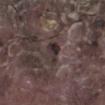Captured during whole-body skin photography for melanoma surveillance; the lesion was not biopsied.
A 15 mm crop from a total-body photograph taken for skin-cancer surveillance.
Located on the left lower leg.
Measured at roughly 3 mm in maximum diameter.
The patient is a male aged around 75.